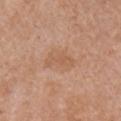Clinical impression: The lesion was tiled from a total-body skin photograph and was not biopsied. Acquisition and patient details: The lesion is located on the right upper arm. A 15 mm crop from a total-body photograph taken for skin-cancer surveillance. The subject is a female about 60 years old.